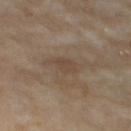Findings:
– follow-up · catalogued during a skin exam; not biopsied
– site · the left thigh
– lesion size · about 2.5 mm
– tile lighting · cross-polarized illumination
– patient · female, about 75 years old
– image source · ~15 mm crop, total-body skin-cancer survey
– automated metrics · a lesion area of about 3.5 mm² and a shape eccentricity near 0.8; a border-irregularity index near 2/10